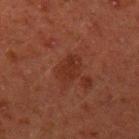Assessment:
Captured during whole-body skin photography for melanoma surveillance; the lesion was not biopsied.
Clinical summary:
Captured under cross-polarized illumination. A 15 mm close-up tile from a total-body photography series done for melanoma screening. The lesion is located on the left upper arm. The total-body-photography lesion software estimated a shape-asymmetry score of about 0.3 (0 = symmetric). And it measured an automated nevus-likeness rating near 0 out of 100 and a lesion-detection confidence of about 100/100. A male patient, about 45 years old. About 4 mm across.